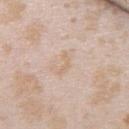workup: no biopsy performed (imaged during a skin exam); lesion diameter: ≈3 mm; anatomic site: the back; image-analysis metrics: border irregularity of about 6 on a 0–10 scale, a color-variation rating of about 0/10, and radial color variation of about 0; subject: female, approximately 25 years of age; acquisition: total-body-photography crop, ~15 mm field of view; tile lighting: white-light.